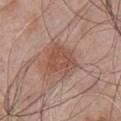biopsy_status: not biopsied; imaged during a skin examination
patient:
  sex: male
  age_approx: 55
lighting: white-light
image:
  source: total-body photography crop
  field_of_view_mm: 15
lesion_size:
  long_diameter_mm_approx: 4.0
site: chest